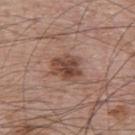Clinical impression: Part of a total-body skin-imaging series; this lesion was reviewed on a skin check and was not flagged for biopsy. Clinical summary: A male patient about 65 years old. The lesion is located on the back. This image is a 15 mm lesion crop taken from a total-body photograph.